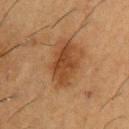The lesion was tiled from a total-body skin photograph and was not biopsied. This image is a 15 mm lesion crop taken from a total-body photograph. Automated image analysis of the tile measured an area of roughly 10 mm², an outline eccentricity of about 0.85 (0 = round, 1 = elongated), and a shape-asymmetry score of about 0.25 (0 = symmetric). The software also gave a lesion color around L≈38 a*≈20 b*≈32 in CIELAB, roughly 9 lightness units darker than nearby skin, and a lesion-to-skin contrast of about 8.5 (normalized; higher = more distinct). Approximately 5 mm at its widest. A male subject, in their mid- to late 50s. Imaged with cross-polarized lighting. The lesion is located on the upper back.